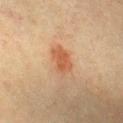Clinical impression: The lesion was tiled from a total-body skin photograph and was not biopsied. Image and clinical context: The lesion is located on the front of the torso. Longest diameter approximately 4 mm. This image is a 15 mm lesion crop taken from a total-body photograph. Automated tile analysis of the lesion measured a footprint of about 7 mm², an outline eccentricity of about 0.85 (0 = round, 1 = elongated), and a symmetry-axis asymmetry near 0.15. The software also gave roughly 9 lightness units darker than nearby skin and a lesion-to-skin contrast of about 7 (normalized; higher = more distinct). The analysis additionally found a nevus-likeness score of about 75/100 and a lesion-detection confidence of about 100/100. A male patient, in their 40s.